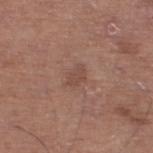<case>
  <biopsy_status>not biopsied; imaged during a skin examination</biopsy_status>
  <lesion_size>
    <long_diameter_mm_approx>2.5</long_diameter_mm_approx>
  </lesion_size>
  <patient>
    <sex>male</sex>
    <age_approx>65</age_approx>
  </patient>
  <lighting>white-light</lighting>
  <site>leg</site>
  <automated_metrics>
    <area_mm2_approx>3.0</area_mm2_approx>
    <eccentricity>0.75</eccentricity>
    <shape_asymmetry>0.3</shape_asymmetry>
    <cielab_L>47</cielab_L>
    <cielab_a>20</cielab_a>
    <cielab_b>26</cielab_b>
    <vs_skin_darker_L>7.0</vs_skin_darker_L>
  </automated_metrics>
  <image>
    <source>total-body photography crop</source>
    <field_of_view_mm>15</field_of_view_mm>
  </image>
</case>